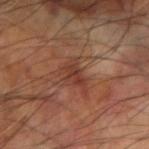Q: How was this image acquired?
A: 15 mm crop, total-body photography
Q: Automated lesion metrics?
A: about 7 CIELAB-L* units darker than the surrounding skin and a normalized lesion–skin contrast near 6.5; a classifier nevus-likeness of about 0/100
Q: Patient demographics?
A: male, about 60 years old
Q: Lesion location?
A: the left arm